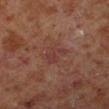<lesion>
  <biopsy_status>not biopsied; imaged during a skin examination</biopsy_status>
  <site>left lower leg</site>
  <image>
    <source>total-body photography crop</source>
    <field_of_view_mm>15</field_of_view_mm>
  </image>
  <lighting>cross-polarized</lighting>
  <automated_metrics>
    <area_mm2_approx>5.5</area_mm2_approx>
    <eccentricity>0.65</eccentricity>
    <vs_skin_darker_L>5.0</vs_skin_darker_L>
    <vs_skin_contrast_norm>5.0</vs_skin_contrast_norm>
    <nevus_likeness_0_100>0</nevus_likeness_0_100>
  </automated_metrics>
  <lesion_size>
    <long_diameter_mm_approx>3.0</long_diameter_mm_approx>
  </lesion_size>
  <patient>
    <sex>male</sex>
    <age_approx>60</age_approx>
  </patient>
</lesion>